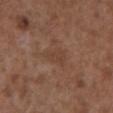Q: Was this lesion biopsied?
A: total-body-photography surveillance lesion; no biopsy
Q: Patient demographics?
A: male, in their mid-70s
Q: How was this image acquired?
A: total-body-photography crop, ~15 mm field of view
Q: How was the tile lit?
A: white-light illumination
Q: Lesion size?
A: ≈3.5 mm
Q: What is the anatomic site?
A: the front of the torso